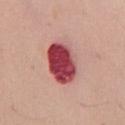<lesion>
<biopsy_status>not biopsied; imaged during a skin examination</biopsy_status>
<patient>
  <sex>female</sex>
  <age_approx>65</age_approx>
</patient>
<site>chest</site>
<image>
  <source>total-body photography crop</source>
  <field_of_view_mm>15</field_of_view_mm>
</image>
</lesion>